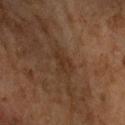Part of a total-body skin-imaging series; this lesion was reviewed on a skin check and was not flagged for biopsy.
An algorithmic analysis of the crop reported an area of roughly 6 mm².
The lesion is on the left upper arm.
This is a cross-polarized tile.
A lesion tile, about 15 mm wide, cut from a 3D total-body photograph.
A female patient aged 58–62.
The recorded lesion diameter is about 4 mm.